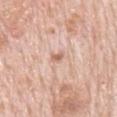Imaged with white-light lighting. Cropped from a total-body skin-imaging series; the visible field is about 15 mm. The lesion is located on the back. Approximately 2.5 mm at its widest. A male subject in their 80s.